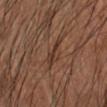biopsy status = total-body-photography surveillance lesion; no biopsy | diameter = ≈3 mm | image = total-body-photography crop, ~15 mm field of view | site = the left forearm | subject = male, in their mid- to late 50s | lighting = cross-polarized.